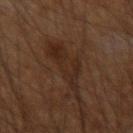biopsy status: catalogued during a skin exam; not biopsied
lesion diameter: ~7 mm (longest diameter)
patient: male, roughly 60 years of age
body site: the left forearm
acquisition: ~15 mm tile from a whole-body skin photo